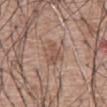Clinical summary: A male patient, aged 58 to 62. Cropped from a whole-body photographic skin survey; the tile spans about 15 mm. From the abdomen. Measured at roughly 3.5 mm in maximum diameter. Captured under white-light illumination. Automated tile analysis of the lesion measured peripheral color asymmetry of about 1. The analysis additionally found an automated nevus-likeness rating near 10 out of 100.